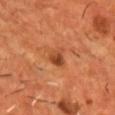{
  "biopsy_status": "not biopsied; imaged during a skin examination",
  "lighting": "cross-polarized",
  "patient": {
    "sex": "male",
    "age_approx": 55
  },
  "image": {
    "source": "total-body photography crop",
    "field_of_view_mm": 15
  },
  "site": "chest",
  "lesion_size": {
    "long_diameter_mm_approx": 2.5
  }
}